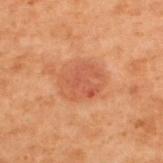Imaged during a routine full-body skin examination; the lesion was not biopsied and no histopathology is available. A 15 mm close-up extracted from a 3D total-body photography capture. The recorded lesion diameter is about 4.5 mm. A male subject, aged approximately 70. The lesion is on the upper back.